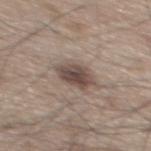Findings:
- biopsy status — imaged on a skin check; not biopsied
- image-analysis metrics — a mean CIELAB color near L≈47 a*≈13 b*≈21, a lesion–skin lightness drop of about 12, and a normalized border contrast of about 9; a border-irregularity rating of about 2/10 and a color-variation rating of about 4.5/10
- anatomic site — the mid back
- illumination — white-light
- size — about 4 mm
- image source — ~15 mm crop, total-body skin-cancer survey
- subject — male, aged 73–77